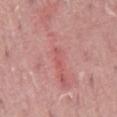Findings:
- workup: total-body-photography surveillance lesion; no biopsy
- image: ~15 mm crop, total-body skin-cancer survey
- body site: the abdomen
- subject: male, in their 40s